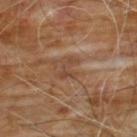<tbp_lesion>
  <biopsy_status>not biopsied; imaged during a skin examination</biopsy_status>
  <site>chest</site>
  <image>
    <source>total-body photography crop</source>
    <field_of_view_mm>15</field_of_view_mm>
  </image>
  <lesion_size>
    <long_diameter_mm_approx>2.5</long_diameter_mm_approx>
  </lesion_size>
  <patient>
    <sex>male</sex>
    <age_approx>60</age_approx>
  </patient>
</tbp_lesion>